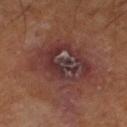Q: Was a biopsy performed?
A: imaged on a skin check; not biopsied
Q: What did automated image analysis measure?
A: a footprint of about 28 mm², an eccentricity of roughly 0.6, and a shape-asymmetry score of about 0.2 (0 = symmetric); a mean CIELAB color near L≈33 a*≈20 b*≈19, a lesion–skin lightness drop of about 7, and a normalized border contrast of about 9; a border-irregularity index near 3/10, a color-variation rating of about 8.5/10, and peripheral color asymmetry of about 2.5; a classifier nevus-likeness of about 0/100 and lesion-presence confidence of about 100/100
Q: Illumination type?
A: cross-polarized illumination
Q: How large is the lesion?
A: ≈6.5 mm
Q: How was this image acquired?
A: ~15 mm crop, total-body skin-cancer survey
Q: Patient demographics?
A: male, aged approximately 60
Q: What is the anatomic site?
A: the right lower leg